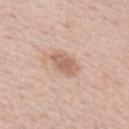biopsy status: no biopsy performed (imaged during a skin exam)
size: ~3 mm (longest diameter)
body site: the left upper arm
imaging modality: ~15 mm tile from a whole-body skin photo
tile lighting: white-light illumination
subject: female, aged 38–42